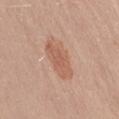A female subject, aged 28–32. From the right thigh. Captured under white-light illumination. A 15 mm crop from a total-body photograph taken for skin-cancer surveillance.